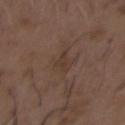Q: Was a biopsy performed?
A: no biopsy performed (imaged during a skin exam)
Q: What are the patient's age and sex?
A: male, aged approximately 50
Q: Lesion location?
A: the front of the torso
Q: How was this image acquired?
A: 15 mm crop, total-body photography